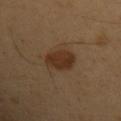Image and clinical context: Located on the left upper arm. Cropped from a total-body skin-imaging series; the visible field is about 15 mm. The patient is a male aged around 55. An algorithmic analysis of the crop reported a mean CIELAB color near L≈30 a*≈17 b*≈27 and a lesion-to-skin contrast of about 9.5 (normalized; higher = more distinct).